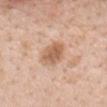Cropped from a total-body skin-imaging series; the visible field is about 15 mm.
An algorithmic analysis of the crop reported a shape-asymmetry score of about 0.2 (0 = symmetric). And it measured an average lesion color of about L≈61 a*≈21 b*≈32 (CIELAB), about 11 CIELAB-L* units darker than the surrounding skin, and a normalized lesion–skin contrast near 7.5. The software also gave border irregularity of about 2 on a 0–10 scale, a within-lesion color-variation index near 3/10, and peripheral color asymmetry of about 1. And it measured a classifier nevus-likeness of about 85/100.
Approximately 3.5 mm at its widest.
Imaged with white-light lighting.
From the mid back.
A female subject, aged around 50.